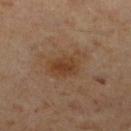Q: Was a biopsy performed?
A: total-body-photography surveillance lesion; no biopsy
Q: How was this image acquired?
A: total-body-photography crop, ~15 mm field of view
Q: Where on the body is the lesion?
A: the right lower leg
Q: Patient demographics?
A: male, aged 58–62
Q: How large is the lesion?
A: about 5 mm